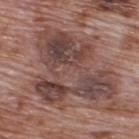Case summary:
- biopsy status — total-body-photography surveillance lesion; no biopsy
- subject — male, about 70 years old
- location — the upper back
- image — ~15 mm crop, total-body skin-cancer survey